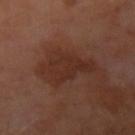– workup · catalogued during a skin exam; not biopsied
– patient · male, aged approximately 50
– location · the left arm
– image · 15 mm crop, total-body photography
– lesion diameter · ≈5.5 mm
– tile lighting · cross-polarized